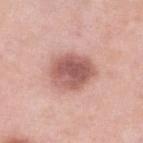Q: Was this lesion biopsied?
A: catalogued during a skin exam; not biopsied
Q: What did automated image analysis measure?
A: an eccentricity of roughly 0.7 and a symmetry-axis asymmetry near 0.15; a border-irregularity index near 1.5/10, internal color variation of about 5 on a 0–10 scale, and a peripheral color-asymmetry measure near 1.5
Q: What lighting was used for the tile?
A: white-light
Q: How was this image acquired?
A: ~15 mm tile from a whole-body skin photo
Q: How large is the lesion?
A: ≈5 mm
Q: Who is the patient?
A: female, in their 40s
Q: Lesion location?
A: the left lower leg